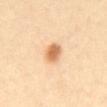Captured during whole-body skin photography for melanoma surveillance; the lesion was not biopsied.
The tile uses cross-polarized illumination.
The lesion is located on the mid back.
About 3 mm across.
Automated tile analysis of the lesion measured a footprint of about 5 mm² and an eccentricity of roughly 0.8. And it measured a detector confidence of about 100 out of 100 that the crop contains a lesion.
The patient is a female aged around 40.
A 15 mm crop from a total-body photograph taken for skin-cancer surveillance.